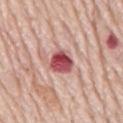No biopsy was performed on this lesion — it was imaged during a full skin examination and was not determined to be concerning. The recorded lesion diameter is about 3.5 mm. The lesion is located on the mid back. Cropped from a whole-body photographic skin survey; the tile spans about 15 mm. The subject is a male aged around 80.